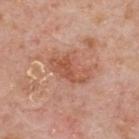  biopsy_status: not biopsied; imaged during a skin examination